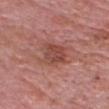Case summary:
– notes — imaged on a skin check; not biopsied
– image — 15 mm crop, total-body photography
– body site — the head or neck
– subject — male, aged approximately 60
– illumination — white-light
– size — ≈4.5 mm
– automated metrics — a footprint of about 10 mm², a shape eccentricity near 0.75, and a symmetry-axis asymmetry near 0.25; an average lesion color of about L≈47 a*≈25 b*≈26 (CIELAB), about 9 CIELAB-L* units darker than the surrounding skin, and a lesion-to-skin contrast of about 6.5 (normalized; higher = more distinct); border irregularity of about 3 on a 0–10 scale and radial color variation of about 1; a detector confidence of about 100 out of 100 that the crop contains a lesion